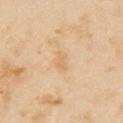Assessment:
No biopsy was performed on this lesion — it was imaged during a full skin examination and was not determined to be concerning.
Background:
The subject is a female roughly 35 years of age. A close-up tile cropped from a whole-body skin photograph, about 15 mm across. From the right upper arm.